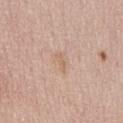Impression: The lesion was tiled from a total-body skin photograph and was not biopsied. Context: Captured under white-light illumination. From the abdomen. The patient is a male roughly 60 years of age. A region of skin cropped from a whole-body photographic capture, roughly 15 mm wide. Longest diameter approximately 2.5 mm.